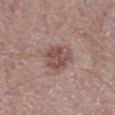Imaged during a routine full-body skin examination; the lesion was not biopsied and no histopathology is available. A 15 mm crop from a total-body photograph taken for skin-cancer surveillance. The subject is a male in their mid- to late 60s. The lesion is located on the right lower leg.